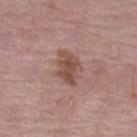* notes: total-body-photography surveillance lesion; no biopsy
* lighting: white-light illumination
* lesion size: ≈4 mm
* TBP lesion metrics: an area of roughly 8.5 mm², an eccentricity of roughly 0.75, and a shape-asymmetry score of about 0.2 (0 = symmetric); a detector confidence of about 100 out of 100 that the crop contains a lesion
* acquisition: 15 mm crop, total-body photography
* subject: female, aged 68 to 72
* body site: the left lower leg